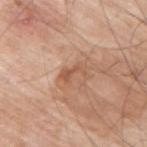notes — imaged on a skin check; not biopsied
illumination — white-light illumination
diameter — about 3 mm
patient — male, aged 68 to 72
acquisition — ~15 mm crop, total-body skin-cancer survey
site — the upper back